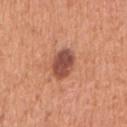Imaged during a routine full-body skin examination; the lesion was not biopsied and no histopathology is available.
Imaged with white-light lighting.
The patient is a female roughly 55 years of age.
On the right upper arm.
This image is a 15 mm lesion crop taken from a total-body photograph.
The lesion's longest dimension is about 3.5 mm.